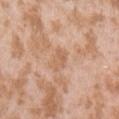notes=total-body-photography surveillance lesion; no biopsy
tile lighting=white-light
anatomic site=the left upper arm
subject=female, roughly 25 years of age
acquisition=~15 mm tile from a whole-body skin photo
TBP lesion metrics=an average lesion color of about L≈61 a*≈20 b*≈33 (CIELAB) and about 7 CIELAB-L* units darker than the surrounding skin; a color-variation rating of about 1.5/10 and radial color variation of about 0.5
lesion diameter=≈3 mm